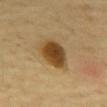<case>
  <biopsy_status>not biopsied; imaged during a skin examination</biopsy_status>
  <site>mid back</site>
  <automated_metrics>
    <area_mm2_approx>12.0</area_mm2_approx>
    <nevus_likeness_0_100>100</nevus_likeness_0_100>
    <lesion_detection_confidence_0_100>100</lesion_detection_confidence_0_100>
  </automated_metrics>
  <patient>
    <sex>male</sex>
    <age_approx>65</age_approx>
  </patient>
  <lighting>cross-polarized</lighting>
  <image>
    <source>total-body photography crop</source>
    <field_of_view_mm>15</field_of_view_mm>
  </image>
  <lesion_size>
    <long_diameter_mm_approx>4.5</long_diameter_mm_approx>
  </lesion_size>
</case>